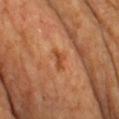This is a cross-polarized tile.
Automated image analysis of the tile measured an eccentricity of roughly 0.85 and two-axis asymmetry of about 0.45. The software also gave border irregularity of about 4.5 on a 0–10 scale, a within-lesion color-variation index near 0.5/10, and a peripheral color-asymmetry measure near 0.
A male subject, about 65 years old.
Cropped from a total-body skin-imaging series; the visible field is about 15 mm.
The lesion is located on the chest.
Measured at roughly 2.5 mm in maximum diameter.